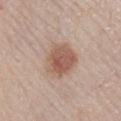The lesion was photographed on a routine skin check and not biopsied; there is no pathology result.
This is a white-light tile.
From the right lower leg.
A region of skin cropped from a whole-body photographic capture, roughly 15 mm wide.
A male subject, aged 58–62.